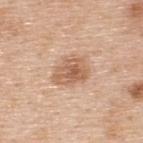* workup · total-body-photography surveillance lesion; no biopsy
* lesion diameter · ≈4 mm
* patient · male, about 50 years old
* imaging modality · total-body-photography crop, ~15 mm field of view
* anatomic site · the upper back